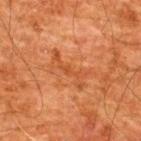anatomic site — the upper back; image source — ~15 mm crop, total-body skin-cancer survey; subject — male, aged approximately 60.